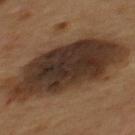Imaged during a routine full-body skin examination; the lesion was not biopsied and no histopathology is available.
A male subject aged 53–57.
A lesion tile, about 15 mm wide, cut from a 3D total-body photograph.
The lesion's longest dimension is about 11.5 mm.
This is a cross-polarized tile.
From the mid back.
The total-body-photography lesion software estimated an average lesion color of about L≈28 a*≈14 b*≈22 (CIELAB), roughly 14 lightness units darker than nearby skin, and a normalized lesion–skin contrast near 14. The software also gave a peripheral color-asymmetry measure near 1.5. It also reported a nevus-likeness score of about 0/100 and a lesion-detection confidence of about 100/100.